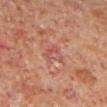A 15 mm crop from a total-body photograph taken for skin-cancer surveillance.
A male subject, aged 58 to 62.
Longest diameter approximately 3 mm.
This is a cross-polarized tile.
On the left lower leg.